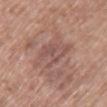Captured during whole-body skin photography for melanoma surveillance; the lesion was not biopsied. A female patient aged around 65. From the chest. A lesion tile, about 15 mm wide, cut from a 3D total-body photograph.